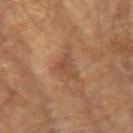Findings:
– tile lighting · cross-polarized
– automated lesion analysis · a mean CIELAB color near L≈47 a*≈21 b*≈32, about 7 CIELAB-L* units darker than the surrounding skin, and a lesion-to-skin contrast of about 5.5 (normalized; higher = more distinct); border irregularity of about 7 on a 0–10 scale, internal color variation of about 3 on a 0–10 scale, and a peripheral color-asymmetry measure near 1
– image source · ~15 mm tile from a whole-body skin photo
– subject · male, aged approximately 65
– size · ≈4 mm
– anatomic site · the left upper arm